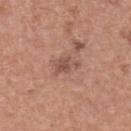workup: catalogued during a skin exam; not biopsied
body site: the upper back
subject: female, approximately 60 years of age
image source: ~15 mm tile from a whole-body skin photo
size: ≈3.5 mm
automated metrics: a lesion color around L≈52 a*≈21 b*≈27 in CIELAB